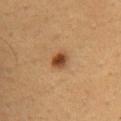Part of a total-body skin-imaging series; this lesion was reviewed on a skin check and was not flagged for biopsy. This image is a 15 mm lesion crop taken from a total-body photograph. A male subject, approximately 45 years of age. An algorithmic analysis of the crop reported a lesion area of about 4 mm², an eccentricity of roughly 0.5, and a shape-asymmetry score of about 0.15 (0 = symmetric). It also reported a lesion color around L≈42 a*≈22 b*≈35 in CIELAB and a lesion–skin lightness drop of about 14. The software also gave lesion-presence confidence of about 100/100. The lesion is located on the chest. Captured under cross-polarized illumination.